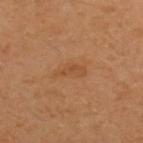Assessment:
Captured during whole-body skin photography for melanoma surveillance; the lesion was not biopsied.
Background:
A male subject, roughly 30 years of age. The lesion is located on the upper back. A lesion tile, about 15 mm wide, cut from a 3D total-body photograph.